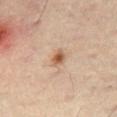| feature | finding |
|---|---|
| workup | imaged on a skin check; not biopsied |
| automated lesion analysis | a mean CIELAB color near L≈55 a*≈20 b*≈33, roughly 12 lightness units darker than nearby skin, and a normalized lesion–skin contrast near 9; a classifier nevus-likeness of about 90/100 and a lesion-detection confidence of about 100/100 |
| image | total-body-photography crop, ~15 mm field of view |
| patient | male, aged approximately 55 |
| body site | the leg |
| lighting | cross-polarized |